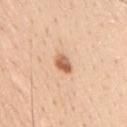Assessment: The lesion was tiled from a total-body skin photograph and was not biopsied. Background: The lesion's longest dimension is about 2.5 mm. A close-up tile cropped from a whole-body skin photograph, about 15 mm across. The patient is a male in their 30s. This is a white-light tile. The lesion is on the mid back.